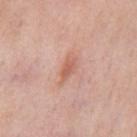Clinical impression: Imaged during a routine full-body skin examination; the lesion was not biopsied and no histopathology is available. Image and clinical context: Located on the chest. A 15 mm close-up tile from a total-body photography series done for melanoma screening. The patient is a male about 55 years old.